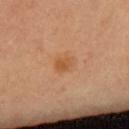Assessment: This lesion was catalogued during total-body skin photography and was not selected for biopsy. Clinical summary: A 15 mm close-up tile from a total-body photography series done for melanoma screening. The lesion-visualizer software estimated a footprint of about 4 mm², an outline eccentricity of about 0.65 (0 = round, 1 = elongated), and a symmetry-axis asymmetry near 0.2. It also reported a color-variation rating of about 2.5/10 and radial color variation of about 1. The analysis additionally found an automated nevus-likeness rating near 50 out of 100. This is a cross-polarized tile. The recorded lesion diameter is about 2.5 mm. A female subject aged 48 to 52.